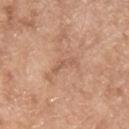| key | value |
|---|---|
| body site | the chest |
| subject | male, in their mid-60s |
| size | ≈3.5 mm |
| imaging modality | ~15 mm crop, total-body skin-cancer survey |
| tile lighting | white-light illumination |
| automated metrics | internal color variation of about 0 on a 0–10 scale |